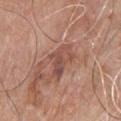Impression: Recorded during total-body skin imaging; not selected for excision or biopsy. Clinical summary: On the chest. A 15 mm close-up tile from a total-body photography series done for melanoma screening. A male subject in their 80s.